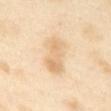No biopsy was performed on this lesion — it was imaged during a full skin examination and was not determined to be concerning. Measured at roughly 5 mm in maximum diameter. A female subject aged 53 to 57. A 15 mm close-up tile from a total-body photography series done for melanoma screening. Located on the abdomen. The lesion-visualizer software estimated a mean CIELAB color near L≈76 a*≈15 b*≈39, about 9 CIELAB-L* units darker than the surrounding skin, and a lesion-to-skin contrast of about 5.5 (normalized; higher = more distinct). And it measured a nevus-likeness score of about 5/100 and lesion-presence confidence of about 100/100. Captured under cross-polarized illumination.